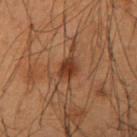| field | value |
|---|---|
| workup | total-body-photography surveillance lesion; no biopsy |
| illumination | cross-polarized |
| image source | ~15 mm tile from a whole-body skin photo |
| size | about 3 mm |
| subject | male, aged 53–57 |
| location | the right forearm |
| TBP lesion metrics | an average lesion color of about L≈30 a*≈19 b*≈27 (CIELAB), a lesion–skin lightness drop of about 9, and a lesion-to-skin contrast of about 9 (normalized; higher = more distinct); a nevus-likeness score of about 90/100 and a detector confidence of about 100 out of 100 that the crop contains a lesion |